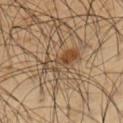Q: Lesion size?
A: about 4.5 mm
Q: How was the tile lit?
A: cross-polarized illumination
Q: What are the patient's age and sex?
A: male, approximately 50 years of age
Q: Lesion location?
A: the arm
Q: What did automated image analysis measure?
A: a footprint of about 8 mm², an outline eccentricity of about 0.9 (0 = round, 1 = elongated), and two-axis asymmetry of about 0.4; an average lesion color of about L≈44 a*≈15 b*≈31 (CIELAB); a border-irregularity index near 7/10; a classifier nevus-likeness of about 75/100 and a lesion-detection confidence of about 95/100
Q: What is the imaging modality?
A: ~15 mm crop, total-body skin-cancer survey